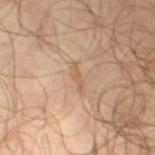Q: Was a biopsy performed?
A: catalogued during a skin exam; not biopsied
Q: What lighting was used for the tile?
A: cross-polarized illumination
Q: How large is the lesion?
A: about 3.5 mm
Q: What are the patient's age and sex?
A: male, aged 63 to 67
Q: How was this image acquired?
A: ~15 mm tile from a whole-body skin photo
Q: Lesion location?
A: the leg
Q: Automated lesion metrics?
A: an outline eccentricity of about 0.95 (0 = round, 1 = elongated) and a shape-asymmetry score of about 0.35 (0 = symmetric); border irregularity of about 4.5 on a 0–10 scale, a color-variation rating of about 0/10, and radial color variation of about 0; a nevus-likeness score of about 0/100